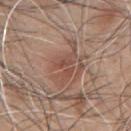notes = imaged on a skin check; not biopsied | anatomic site = the left upper arm | patient = male, aged around 45 | acquisition = 15 mm crop, total-body photography.